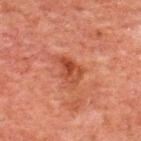biopsy status = catalogued during a skin exam; not biopsied | anatomic site = the upper back | imaging modality = total-body-photography crop, ~15 mm field of view | size = ~3 mm (longest diameter) | subject = male, approximately 60 years of age.